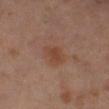Impression: This lesion was catalogued during total-body skin photography and was not selected for biopsy. Acquisition and patient details: Imaged with cross-polarized lighting. An algorithmic analysis of the crop reported an average lesion color of about L≈40 a*≈19 b*≈27 (CIELAB), about 6 CIELAB-L* units darker than the surrounding skin, and a normalized lesion–skin contrast near 6.5. The patient is a male aged around 60. The lesion's longest dimension is about 3 mm. Cropped from a whole-body photographic skin survey; the tile spans about 15 mm. The lesion is located on the right lower leg.